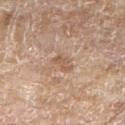Located on the right upper arm. Captured under white-light illumination. The recorded lesion diameter is about 2.5 mm. A 15 mm crop from a total-body photograph taken for skin-cancer surveillance. A male patient about 80 years old.